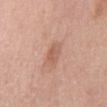Context:
Longest diameter approximately 2.5 mm. A close-up tile cropped from a whole-body skin photograph, about 15 mm across. A male subject approximately 70 years of age. From the mid back. Automated image analysis of the tile measured a lesion color around L≈59 a*≈23 b*≈31 in CIELAB, roughly 8 lightness units darker than nearby skin, and a normalized border contrast of about 5.5. It also reported a border-irregularity rating of about 1.5/10, internal color variation of about 2.5 on a 0–10 scale, and a peripheral color-asymmetry measure near 1. The tile uses white-light illumination.